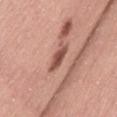The lesion was photographed on a routine skin check and not biopsied; there is no pathology result.